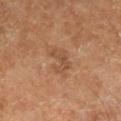Impression: Imaged during a routine full-body skin examination; the lesion was not biopsied and no histopathology is available. Background: A lesion tile, about 15 mm wide, cut from a 3D total-body photograph. The recorded lesion diameter is about 3 mm. Captured under cross-polarized illumination. A male subject, aged approximately 60. On the right lower leg.